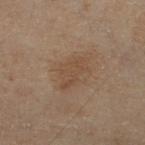  biopsy_status: not biopsied; imaged during a skin examination
  lighting: cross-polarized
  patient:
    sex: male
    age_approx: 70
  lesion_size:
    long_diameter_mm_approx: 4.0
  site: right lower leg
  image:
    source: total-body photography crop
    field_of_view_mm: 15